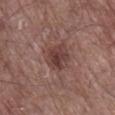Clinical impression: Captured during whole-body skin photography for melanoma surveillance; the lesion was not biopsied. Clinical summary: A roughly 15 mm field-of-view crop from a total-body skin photograph. The patient is a male roughly 80 years of age. On the right lower leg. The recorded lesion diameter is about 3.5 mm. Captured under white-light illumination.